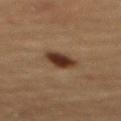The lesion was photographed on a routine skin check and not biopsied; there is no pathology result.
Captured under cross-polarized illumination.
A female subject aged 68 to 72.
From the mid back.
A 15 mm close-up tile from a total-body photography series done for melanoma screening.
The total-body-photography lesion software estimated a lesion area of about 7.5 mm², an eccentricity of roughly 0.6, and a shape-asymmetry score of about 0.2 (0 = symmetric). It also reported an average lesion color of about L≈29 a*≈16 b*≈24 (CIELAB), about 12 CIELAB-L* units darker than the surrounding skin, and a normalized lesion–skin contrast near 12. And it measured a border-irregularity index near 1.5/10, a color-variation rating of about 4/10, and radial color variation of about 1. And it measured a classifier nevus-likeness of about 100/100 and lesion-presence confidence of about 100/100.
Measured at roughly 3.5 mm in maximum diameter.